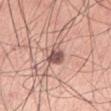| field | value |
|---|---|
| patient | male, approximately 25 years of age |
| tile lighting | white-light |
| acquisition | total-body-photography crop, ~15 mm field of view |
| site | the left upper arm |